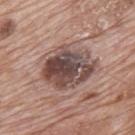Automated tile analysis of the lesion measured a mean CIELAB color near L≈47 a*≈17 b*≈20. The lesion's longest dimension is about 6.5 mm. This image is a 15 mm lesion crop taken from a total-body photograph. From the mid back. The subject is a male aged 68 to 72.